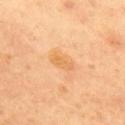Recorded during total-body skin imaging; not selected for excision or biopsy.
A lesion tile, about 15 mm wide, cut from a 3D total-body photograph.
This is a cross-polarized tile.
A female patient, aged approximately 40.
Located on the upper back.
Approximately 3 mm at its widest.